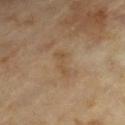No biopsy was performed on this lesion — it was imaged during a full skin examination and was not determined to be concerning.
A male patient aged around 65.
A region of skin cropped from a whole-body photographic capture, roughly 15 mm wide.
Captured under cross-polarized illumination.